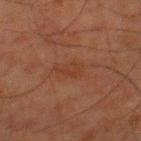Captured during whole-body skin photography for melanoma surveillance; the lesion was not biopsied. A male patient aged approximately 80. A 15 mm close-up tile from a total-body photography series done for melanoma screening. The lesion is located on the right thigh.